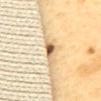Clinical impression: The lesion was photographed on a routine skin check and not biopsied; there is no pathology result. Image and clinical context: Measured at roughly 3 mm in maximum diameter. A region of skin cropped from a whole-body photographic capture, roughly 15 mm wide. An algorithmic analysis of the crop reported a shape-asymmetry score of about 0.4 (0 = symmetric). The software also gave a mean CIELAB color near L≈66 a*≈16 b*≈39, a lesion–skin lightness drop of about 17, and a normalized border contrast of about 10. The analysis additionally found peripheral color asymmetry of about 1.5. And it measured a lesion-detection confidence of about 90/100. The lesion is on the mid back. The tile uses cross-polarized illumination. A female subject aged approximately 55.